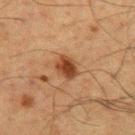{"biopsy_status": "not biopsied; imaged during a skin examination", "image": {"source": "total-body photography crop", "field_of_view_mm": 15}, "automated_metrics": {"border_irregularity_0_10": 2.0, "peripheral_color_asymmetry": 1.0, "nevus_likeness_0_100": 95, "lesion_detection_confidence_0_100": 100}, "patient": {"sex": "male", "age_approx": 60}, "site": "back", "lighting": "cross-polarized"}